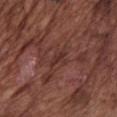| feature | finding |
|---|---|
| biopsy status | no biopsy performed (imaged during a skin exam) |
| lighting | white-light illumination |
| subject | male, aged approximately 75 |
| acquisition | total-body-photography crop, ~15 mm field of view |
| automated lesion analysis | a lesion area of about 2 mm² and two-axis asymmetry of about 0.25; roughly 7 lightness units darker than nearby skin and a lesion-to-skin contrast of about 6.5 (normalized; higher = more distinct); a border-irregularity rating of about 3/10, internal color variation of about 0 on a 0–10 scale, and a peripheral color-asymmetry measure near 0 |
| location | the chest |
| size | about 2.5 mm |